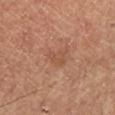{
  "biopsy_status": "not biopsied; imaged during a skin examination",
  "image": {
    "source": "total-body photography crop",
    "field_of_view_mm": 15
  },
  "patient": {
    "sex": "male",
    "age_approx": 65
  },
  "site": "right lower leg",
  "lesion_size": {
    "long_diameter_mm_approx": 2.5
  }
}